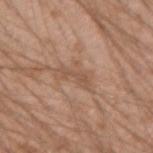Findings:
– follow-up · imaged on a skin check; not biopsied
– patient · male, approximately 20 years of age
– image · total-body-photography crop, ~15 mm field of view
– body site · the right forearm
– image-analysis metrics · a footprint of about 3 mm², a shape eccentricity near 0.95, and a shape-asymmetry score of about 0.55 (0 = symmetric); an average lesion color of about L≈52 a*≈19 b*≈29 (CIELAB), about 7 CIELAB-L* units darker than the surrounding skin, and a lesion-to-skin contrast of about 5 (normalized; higher = more distinct); a classifier nevus-likeness of about 0/100 and a detector confidence of about 95 out of 100 that the crop contains a lesion
– tile lighting · white-light illumination